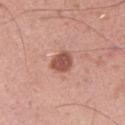Case summary:
• image-analysis metrics: a lesion area of about 5.5 mm² and an outline eccentricity of about 0.6 (0 = round, 1 = elongated); an automated nevus-likeness rating near 70 out of 100 and a lesion-detection confidence of about 100/100
• patient: male, in their mid- to late 30s
• imaging modality: 15 mm crop, total-body photography
• anatomic site: the left upper arm
• diameter: ~3 mm (longest diameter)
• lighting: white-light illumination